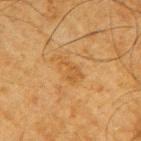Imaged during a routine full-body skin examination; the lesion was not biopsied and no histopathology is available. A male subject, roughly 65 years of age. Located on the right upper arm. Imaged with cross-polarized lighting. A roughly 15 mm field-of-view crop from a total-body skin photograph.